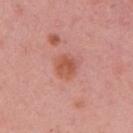Captured during whole-body skin photography for melanoma surveillance; the lesion was not biopsied. The lesion is on the right upper arm. Cropped from a total-body skin-imaging series; the visible field is about 15 mm. The tile uses white-light illumination. About 3 mm across. The lesion-visualizer software estimated a footprint of about 7 mm² and a shape-asymmetry score of about 0.15 (0 = symmetric). The software also gave a lesion color around L≈56 a*≈28 b*≈31 in CIELAB and a normalized lesion–skin contrast near 7. It also reported a nevus-likeness score of about 75/100 and lesion-presence confidence of about 100/100. A female patient, in their mid-30s.